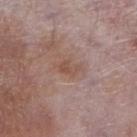This lesion was catalogued during total-body skin photography and was not selected for biopsy. Automated tile analysis of the lesion measured an average lesion color of about L≈51 a*≈19 b*≈26 (CIELAB), roughly 7 lightness units darker than nearby skin, and a normalized lesion–skin contrast near 6. The software also gave a within-lesion color-variation index near 2.5/10 and a peripheral color-asymmetry measure near 1. It also reported a nevus-likeness score of about 0/100 and lesion-presence confidence of about 100/100. Cropped from a whole-body photographic skin survey; the tile spans about 15 mm. The patient is a male about 75 years old. On the right lower leg.